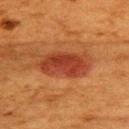Recorded during total-body skin imaging; not selected for excision or biopsy.
The lesion is on the upper back.
Measured at roughly 6 mm in maximum diameter.
A 15 mm crop from a total-body photograph taken for skin-cancer surveillance.
A female patient aged around 50.